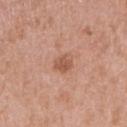The lesion was photographed on a routine skin check and not biopsied; there is no pathology result. Longest diameter approximately 3 mm. Cropped from a whole-body photographic skin survey; the tile spans about 15 mm. A female subject, aged around 30. From the right upper arm.